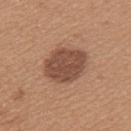Impression: Part of a total-body skin-imaging series; this lesion was reviewed on a skin check and was not flagged for biopsy. Background: A female subject aged 43 to 47. About 5.5 mm across. Automated tile analysis of the lesion measured lesion-presence confidence of about 100/100. The lesion is located on the upper back. Cropped from a total-body skin-imaging series; the visible field is about 15 mm. This is a white-light tile.